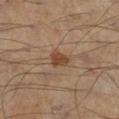imaging modality: ~15 mm crop, total-body skin-cancer survey | patient: male, aged approximately 55 | anatomic site: the leg | illumination: cross-polarized | lesion size: ≈2.5 mm.